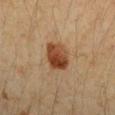Notes:
* biopsy status · imaged on a skin check; not biopsied
* acquisition · total-body-photography crop, ~15 mm field of view
* site · the right forearm
* lesion diameter · ≈3.5 mm
* subject · female, in their 40s